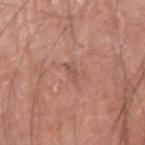Assessment: Part of a total-body skin-imaging series; this lesion was reviewed on a skin check and was not flagged for biopsy. Acquisition and patient details: A 15 mm close-up extracted from a 3D total-body photography capture. A male patient, aged 73–77. On the right upper arm.